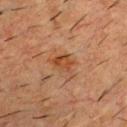The lesion was photographed on a routine skin check and not biopsied; there is no pathology result. The lesion is located on the upper back. The recorded lesion diameter is about 3 mm. A male patient approximately 55 years of age. Cropped from a whole-body photographic skin survey; the tile spans about 15 mm. This is a cross-polarized tile.